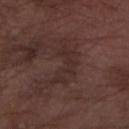Imaged during a routine full-body skin examination; the lesion was not biopsied and no histopathology is available. A male patient approximately 75 years of age. A 15 mm close-up tile from a total-body photography series done for melanoma screening. Located on the arm.